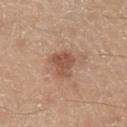biopsy status=total-body-photography surveillance lesion; no biopsy
location=the left lower leg
acquisition=~15 mm crop, total-body skin-cancer survey
lesion diameter=about 3 mm
subject=male, in their 40s